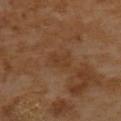biopsy status: no biopsy performed (imaged during a skin exam) | lesion diameter: ≈3 mm | subject: female, approximately 55 years of age | acquisition: ~15 mm tile from a whole-body skin photo | anatomic site: the upper back | lighting: cross-polarized.